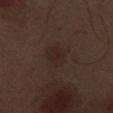{
  "biopsy_status": "not biopsied; imaged during a skin examination"
}